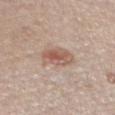Acquisition and patient details: A roughly 15 mm field-of-view crop from a total-body skin photograph. The tile uses white-light illumination. Approximately 4 mm at its widest. The patient is a female aged approximately 55. The lesion is on the chest.